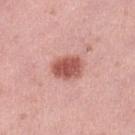Captured during whole-body skin photography for melanoma surveillance; the lesion was not biopsied. Approximately 4 mm at its widest. A female subject, approximately 50 years of age. Automated image analysis of the tile measured a lesion area of about 9 mm² and a shape eccentricity near 0.65. The analysis additionally found border irregularity of about 1.5 on a 0–10 scale, a color-variation rating of about 3.5/10, and a peripheral color-asymmetry measure near 1. And it measured a lesion-detection confidence of about 100/100. Imaged with white-light lighting. On the left lower leg. A 15 mm close-up tile from a total-body photography series done for melanoma screening.